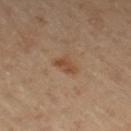  biopsy_status: not biopsied; imaged during a skin examination
  image:
    source: total-body photography crop
    field_of_view_mm: 15
  patient:
    sex: male
    age_approx: 65
  site: leg